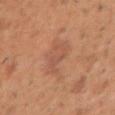Clinical impression:
Captured during whole-body skin photography for melanoma surveillance; the lesion was not biopsied.
Clinical summary:
Measured at roughly 3.5 mm in maximum diameter. A lesion tile, about 15 mm wide, cut from a 3D total-body photograph. Located on the right upper arm. The subject is a male aged 38–42. The tile uses white-light illumination.